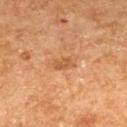Assessment:
Part of a total-body skin-imaging series; this lesion was reviewed on a skin check and was not flagged for biopsy.
Acquisition and patient details:
Cropped from a total-body skin-imaging series; the visible field is about 15 mm. About 2.5 mm across. From the upper back. This is a cross-polarized tile. A female subject, approximately 60 years of age.